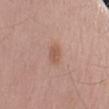Notes:
• workup: total-body-photography surveillance lesion; no biopsy
• body site: the left upper arm
• imaging modality: ~15 mm crop, total-body skin-cancer survey
• patient: male, in their mid- to late 50s
• lighting: white-light
• size: ≈3 mm